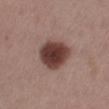Q: Was this lesion biopsied?
A: catalogued during a skin exam; not biopsied
Q: What is the imaging modality?
A: ~15 mm tile from a whole-body skin photo
Q: Illumination type?
A: white-light
Q: What are the patient's age and sex?
A: female, about 50 years old
Q: Where on the body is the lesion?
A: the leg
Q: What is the lesion's diameter?
A: ≈5 mm